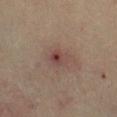The lesion was photographed on a routine skin check and not biopsied; there is no pathology result. Captured under cross-polarized illumination. A 15 mm crop from a total-body photograph taken for skin-cancer surveillance. On the right lower leg. The subject is a male in their mid-60s.